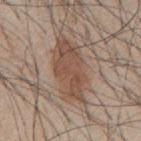workup: imaged on a skin check; not biopsied
patient: male, aged 43–47
size: ≈8 mm
illumination: white-light
image: total-body-photography crop, ~15 mm field of view
anatomic site: the mid back